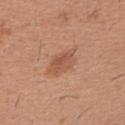Context:
The recorded lesion diameter is about 3.5 mm. A male patient, roughly 40 years of age. A 15 mm close-up extracted from a 3D total-body photography capture. Located on the left upper arm. Captured under white-light illumination.